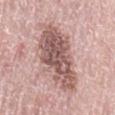{"biopsy_status": "not biopsied; imaged during a skin examination", "lesion_size": {"long_diameter_mm_approx": 8.0}, "image": {"source": "total-body photography crop", "field_of_view_mm": 15}, "site": "left thigh", "patient": {"sex": "female", "age_approx": 60}, "lighting": "white-light"}